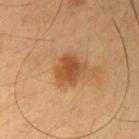Recorded during total-body skin imaging; not selected for excision or biopsy. A lesion tile, about 15 mm wide, cut from a 3D total-body photograph. A male patient roughly 55 years of age. Longest diameter approximately 3.5 mm. The lesion is located on the left upper arm. Automated tile analysis of the lesion measured a lesion area of about 9.5 mm², a shape eccentricity near 0.5, and a symmetry-axis asymmetry near 0.2. And it measured a within-lesion color-variation index near 3.5/10. The software also gave an automated nevus-likeness rating near 95 out of 100 and a detector confidence of about 100 out of 100 that the crop contains a lesion.